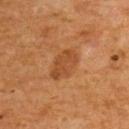| key | value |
|---|---|
| workup | total-body-photography surveillance lesion; no biopsy |
| site | the upper back |
| subject | male, aged 63 to 67 |
| TBP lesion metrics | a classifier nevus-likeness of about 10/100 and lesion-presence confidence of about 100/100 |
| tile lighting | cross-polarized illumination |
| image source | ~15 mm tile from a whole-body skin photo |
| lesion size | ~4 mm (longest diameter) |